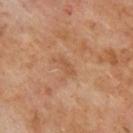Assessment:
Recorded during total-body skin imaging; not selected for excision or biopsy.
Image and clinical context:
The tile uses cross-polarized illumination. From the back. The subject is a male approximately 70 years of age. Automated image analysis of the tile measured two-axis asymmetry of about 0.35. It also reported an average lesion color of about L≈52 a*≈22 b*≈34 (CIELAB), about 6 CIELAB-L* units darker than the surrounding skin, and a lesion-to-skin contrast of about 5 (normalized; higher = more distinct). And it measured a classifier nevus-likeness of about 0/100 and a lesion-detection confidence of about 100/100. Cropped from a total-body skin-imaging series; the visible field is about 15 mm. The lesion's longest dimension is about 2.5 mm.